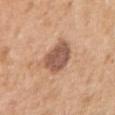Clinical impression:
No biopsy was performed on this lesion — it was imaged during a full skin examination and was not determined to be concerning.
Context:
Cropped from a total-body skin-imaging series; the visible field is about 15 mm. A female subject, aged around 40. The lesion is on the right upper arm. Imaged with white-light lighting. Automated image analysis of the tile measured a footprint of about 11 mm², a shape eccentricity near 0.4, and a symmetry-axis asymmetry near 0.2. The software also gave about 13 CIELAB-L* units darker than the surrounding skin and a normalized lesion–skin contrast near 9. It also reported a border-irregularity index near 2/10, internal color variation of about 2.5 on a 0–10 scale, and a peripheral color-asymmetry measure near 1. The software also gave a classifier nevus-likeness of about 10/100 and lesion-presence confidence of about 100/100. The recorded lesion diameter is about 4 mm.